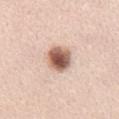{
  "biopsy_status": "not biopsied; imaged during a skin examination",
  "lighting": "white-light",
  "site": "front of the torso",
  "patient": {
    "sex": "male",
    "age_approx": 45
  },
  "image": {
    "source": "total-body photography crop",
    "field_of_view_mm": 15
  },
  "lesion_size": {
    "long_diameter_mm_approx": 4.0
  },
  "automated_metrics": {
    "area_mm2_approx": 9.0,
    "shape_asymmetry": 0.15,
    "nevus_likeness_0_100": 100
  }
}